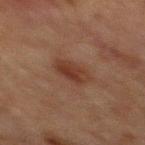follow-up=imaged on a skin check; not biopsied
image=~15 mm tile from a whole-body skin photo
location=the chest
patient=male, about 65 years old